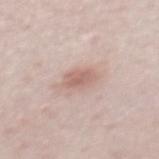| feature | finding |
|---|---|
| biopsy status | imaged on a skin check; not biopsied |
| imaging modality | total-body-photography crop, ~15 mm field of view |
| lesion size | about 3 mm |
| patient | female, aged approximately 50 |
| site | the mid back |
| lighting | white-light illumination |